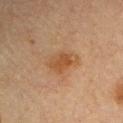Clinical summary:
Automated tile analysis of the lesion measured a footprint of about 8.5 mm², an eccentricity of roughly 0.75, and a shape-asymmetry score of about 0.25 (0 = symmetric). The software also gave a lesion color around L≈41 a*≈17 b*≈30 in CIELAB, a lesion–skin lightness drop of about 6, and a normalized lesion–skin contrast near 7. It also reported an automated nevus-likeness rating near 25 out of 100 and a lesion-detection confidence of about 100/100. A male patient approximately 65 years of age. On the right upper arm. This image is a 15 mm lesion crop taken from a total-body photograph.